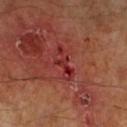The lesion was photographed on a routine skin check and not biopsied; there is no pathology result.
The lesion-visualizer software estimated an average lesion color of about L≈30 a*≈32 b*≈25 (CIELAB), roughly 7 lightness units darker than nearby skin, and a normalized border contrast of about 7.
Located on the left lower leg.
Approximately 4.5 mm at its widest.
A lesion tile, about 15 mm wide, cut from a 3D total-body photograph.
The tile uses cross-polarized illumination.
A male subject aged around 60.